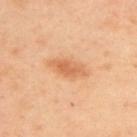A female subject in their 40s. The lesion is on the upper back. A lesion tile, about 15 mm wide, cut from a 3D total-body photograph.